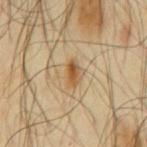notes: catalogued during a skin exam; not biopsied | anatomic site: the back | subject: male, about 65 years old | image source: 15 mm crop, total-body photography | diameter: ≈3 mm | tile lighting: cross-polarized.